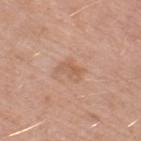Q: Was a biopsy performed?
A: catalogued during a skin exam; not biopsied
Q: What lighting was used for the tile?
A: white-light
Q: What kind of image is this?
A: ~15 mm tile from a whole-body skin photo
Q: Who is the patient?
A: female, in their 70s
Q: What is the anatomic site?
A: the right thigh
Q: How large is the lesion?
A: ~3 mm (longest diameter)